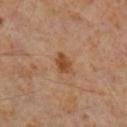Part of a total-body skin-imaging series; this lesion was reviewed on a skin check and was not flagged for biopsy. Located on the leg. A close-up tile cropped from a whole-body skin photograph, about 15 mm across. Imaged with cross-polarized lighting. A male subject, roughly 60 years of age.